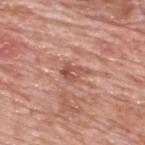Q: Was a biopsy performed?
A: imaged on a skin check; not biopsied
Q: What is the anatomic site?
A: the upper back
Q: How large is the lesion?
A: about 3 mm
Q: What are the patient's age and sex?
A: male, in their 80s
Q: How was the tile lit?
A: white-light illumination
Q: What did automated image analysis measure?
A: a footprint of about 5 mm², an outline eccentricity of about 0.65 (0 = round, 1 = elongated), and a symmetry-axis asymmetry near 0.4; a border-irregularity index near 4/10, a within-lesion color-variation index near 5.5/10, and radial color variation of about 2
Q: How was this image acquired?
A: ~15 mm crop, total-body skin-cancer survey